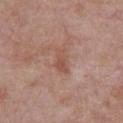{"biopsy_status": "not biopsied; imaged during a skin examination", "image": {"source": "total-body photography crop", "field_of_view_mm": 15}, "lighting": "white-light", "lesion_size": {"long_diameter_mm_approx": 3.5}, "patient": {"sex": "male", "age_approx": 55}, "site": "chest"}